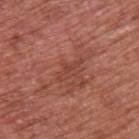The lesion was tiled from a total-body skin photograph and was not biopsied. About 3.5 mm across. A lesion tile, about 15 mm wide, cut from a 3D total-body photograph. The lesion is on the upper back. A male patient, roughly 65 years of age. This is a white-light tile.